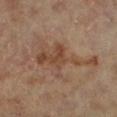Assessment: The lesion was tiled from a total-body skin photograph and was not biopsied. Clinical summary: On the left lower leg. A male patient roughly 85 years of age or older. Cropped from a total-body skin-imaging series; the visible field is about 15 mm. The total-body-photography lesion software estimated a footprint of about 18 mm², a shape eccentricity near 0.9, and a symmetry-axis asymmetry near 0.6. The analysis additionally found a lesion-to-skin contrast of about 6.5 (normalized; higher = more distinct). And it measured a nevus-likeness score of about 0/100 and lesion-presence confidence of about 100/100. Approximately 8.5 mm at its widest. Imaged with cross-polarized lighting.